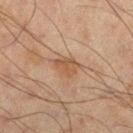workup=imaged on a skin check; not biopsied | lesion size=≈3.5 mm | location=the left lower leg | patient=male, roughly 45 years of age | acquisition=~15 mm tile from a whole-body skin photo.